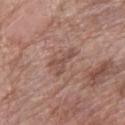Part of a total-body skin-imaging series; this lesion was reviewed on a skin check and was not flagged for biopsy.
A 15 mm close-up extracted from a 3D total-body photography capture.
The lesion is located on the left forearm.
About 3.5 mm across.
A female subject, approximately 60 years of age.
Automated image analysis of the tile measured a lesion color around L≈50 a*≈20 b*≈24 in CIELAB, a lesion–skin lightness drop of about 8, and a lesion-to-skin contrast of about 6 (normalized; higher = more distinct). It also reported a border-irregularity index near 6.5/10 and internal color variation of about 2.5 on a 0–10 scale. The software also gave a nevus-likeness score of about 0/100 and lesion-presence confidence of about 100/100.